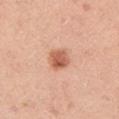Impression:
No biopsy was performed on this lesion — it was imaged during a full skin examination and was not determined to be concerning.
Clinical summary:
Located on the arm. A male subject, aged around 45. A lesion tile, about 15 mm wide, cut from a 3D total-body photograph. Measured at roughly 3 mm in maximum diameter.